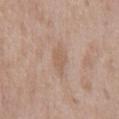Clinical impression:
Imaged during a routine full-body skin examination; the lesion was not biopsied and no histopathology is available.
Acquisition and patient details:
From the chest. The patient is a male about 50 years old. A 15 mm close-up extracted from a 3D total-body photography capture.